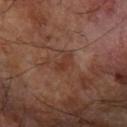Q: Was a biopsy performed?
A: catalogued during a skin exam; not biopsied
Q: Lesion location?
A: the right forearm
Q: Who is the patient?
A: male, aged 63–67
Q: What did automated image analysis measure?
A: a lesion area of about 2.5 mm², an eccentricity of roughly 0.85, and a symmetry-axis asymmetry near 0.35; a lesion-to-skin contrast of about 6 (normalized; higher = more distinct); a nevus-likeness score of about 0/100 and a lesion-detection confidence of about 100/100
Q: What kind of image is this?
A: total-body-photography crop, ~15 mm field of view
Q: How large is the lesion?
A: about 2.5 mm
Q: Illumination type?
A: cross-polarized illumination